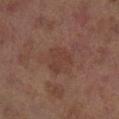This lesion was catalogued during total-body skin photography and was not selected for biopsy. The lesion is on the right lower leg. The subject is a female in their 60s. A 15 mm crop from a total-body photograph taken for skin-cancer surveillance.